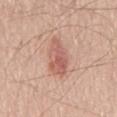  biopsy_status: not biopsied; imaged during a skin examination